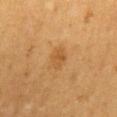follow-up — imaged on a skin check; not biopsied | lesion diameter — ≈2.5 mm | patient — male, about 60 years old | automated lesion analysis — a lesion area of about 3.5 mm² and an outline eccentricity of about 0.75 (0 = round, 1 = elongated); an average lesion color of about L≈53 a*≈22 b*≈42 (CIELAB); a border-irregularity index near 2.5/10 and peripheral color asymmetry of about 0.5 | acquisition — ~15 mm crop, total-body skin-cancer survey | body site — the mid back.